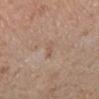Q: Is there a histopathology result?
A: no biopsy performed (imaged during a skin exam)
Q: Who is the patient?
A: male, in their mid-60s
Q: What lighting was used for the tile?
A: white-light
Q: How large is the lesion?
A: about 2.5 mm
Q: Lesion location?
A: the right lower leg
Q: What is the imaging modality?
A: ~15 mm crop, total-body skin-cancer survey